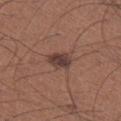Assessment:
This lesion was catalogued during total-body skin photography and was not selected for biopsy.
Context:
On the right lower leg. A 15 mm crop from a total-body photograph taken for skin-cancer surveillance. A male patient in their mid- to late 60s.